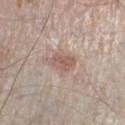Clinical impression: This lesion was catalogued during total-body skin photography and was not selected for biopsy. Context: Located on the right lower leg. Longest diameter approximately 3 mm. The subject is a male roughly 60 years of age. The total-body-photography lesion software estimated a lesion color around L≈57 a*≈18 b*≈23 in CIELAB, roughly 10 lightness units darker than nearby skin, and a normalized border contrast of about 6.5. The analysis additionally found a nevus-likeness score of about 50/100. A region of skin cropped from a whole-body photographic capture, roughly 15 mm wide.